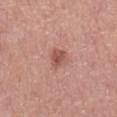Q: Is there a histopathology result?
A: imaged on a skin check; not biopsied
Q: Where on the body is the lesion?
A: the mid back
Q: Who is the patient?
A: male, aged 68 to 72
Q: How was the tile lit?
A: white-light illumination
Q: How was this image acquired?
A: ~15 mm crop, total-body skin-cancer survey